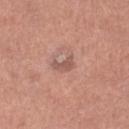Background: A 15 mm close-up tile from a total-body photography series done for melanoma screening. About 2.5 mm across. From the leg. The patient is a female aged around 50. The tile uses white-light illumination.